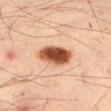| field | value |
|---|---|
| workup | no biopsy performed (imaged during a skin exam) |
| automated lesion analysis | a mean CIELAB color near L≈49 a*≈23 b*≈34, about 20 CIELAB-L* units darker than the surrounding skin, and a normalized border contrast of about 13.5; a border-irregularity index near 1.5/10, a within-lesion color-variation index near 7/10, and a peripheral color-asymmetry measure near 2.5 |
| anatomic site | the leg |
| patient | male, aged around 35 |
| illumination | cross-polarized illumination |
| image | total-body-photography crop, ~15 mm field of view |
| size | about 4.5 mm |